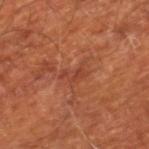Q: Was this lesion biopsied?
A: catalogued during a skin exam; not biopsied
Q: What did automated image analysis measure?
A: a footprint of about 3 mm², an outline eccentricity of about 0.85 (0 = round, 1 = elongated), and a symmetry-axis asymmetry near 0.45; a lesion color around L≈42 a*≈29 b*≈32 in CIELAB, roughly 6 lightness units darker than nearby skin, and a lesion-to-skin contrast of about 5.5 (normalized; higher = more distinct); a border-irregularity index near 5/10, a within-lesion color-variation index near 0/10, and radial color variation of about 0
Q: What are the patient's age and sex?
A: aged 63 to 67
Q: Where on the body is the lesion?
A: the right lower leg
Q: Lesion size?
A: ≈3 mm
Q: How was this image acquired?
A: ~15 mm crop, total-body skin-cancer survey